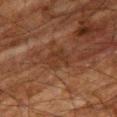No biopsy was performed on this lesion — it was imaged during a full skin examination and was not determined to be concerning. On the right thigh. The patient is a male about 80 years old. The recorded lesion diameter is about 3 mm. Automated image analysis of the tile measured a footprint of about 6.5 mm² and an outline eccentricity of about 0.5 (0 = round, 1 = elongated). The software also gave border irregularity of about 3 on a 0–10 scale and a color-variation rating of about 2/10. It also reported a classifier nevus-likeness of about 0/100 and a lesion-detection confidence of about 95/100. Imaged with cross-polarized lighting. Cropped from a total-body skin-imaging series; the visible field is about 15 mm.